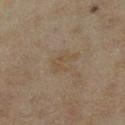notes: no biopsy performed (imaged during a skin exam); lesion size: about 3 mm; acquisition: 15 mm crop, total-body photography; illumination: cross-polarized illumination; TBP lesion metrics: a lesion–skin lightness drop of about 4 and a normalized lesion–skin contrast near 4.5; site: the left lower leg; subject: female, aged 58 to 62.